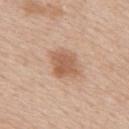workup=no biopsy performed (imaged during a skin exam) | automated lesion analysis=border irregularity of about 2 on a 0–10 scale, a within-lesion color-variation index near 2.5/10, and a peripheral color-asymmetry measure near 1 | site=the upper back | size=about 3.5 mm | patient=male, roughly 60 years of age | acquisition=~15 mm tile from a whole-body skin photo | tile lighting=white-light illumination.